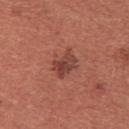Context: A female subject, approximately 35 years of age. This is a white-light tile. Approximately 3 mm at its widest. Located on the chest. A 15 mm close-up tile from a total-body photography series done for melanoma screening.